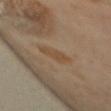Impression: The lesion was tiled from a total-body skin photograph and was not biopsied. Acquisition and patient details: Located on the mid back. A female patient, in their mid-60s. A lesion tile, about 15 mm wide, cut from a 3D total-body photograph.